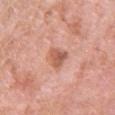The lesion was tiled from a total-body skin photograph and was not biopsied.